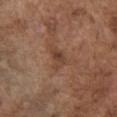Notes:
• follow-up — imaged on a skin check; not biopsied
• lesion diameter — ≈3 mm
• body site — the chest
• acquisition — 15 mm crop, total-body photography
• subject — male, aged 73–77
• illumination — white-light